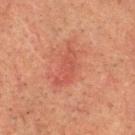Q: Is there a histopathology result?
A: imaged on a skin check; not biopsied
Q: Lesion location?
A: the head or neck
Q: How large is the lesion?
A: about 5.5 mm
Q: What kind of image is this?
A: ~15 mm crop, total-body skin-cancer survey
Q: Patient demographics?
A: male, about 60 years old